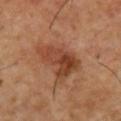| field | value |
|---|---|
| subject | male, about 55 years old |
| location | the chest |
| lighting | cross-polarized |
| diameter | ~5.5 mm (longest diameter) |
| imaging modality | total-body-photography crop, ~15 mm field of view |